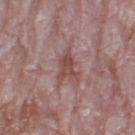No biopsy was performed on this lesion — it was imaged during a full skin examination and was not determined to be concerning. Automated tile analysis of the lesion measured a lesion area of about 5 mm², an eccentricity of roughly 0.85, and a symmetry-axis asymmetry near 0.45. It also reported an average lesion color of about L≈47 a*≈22 b*≈21 (CIELAB) and roughly 9 lightness units darker than nearby skin. The analysis additionally found a border-irregularity index near 5/10, a within-lesion color-variation index near 3/10, and radial color variation of about 1. It also reported a nevus-likeness score of about 0/100 and a lesion-detection confidence of about 70/100. Measured at roughly 3.5 mm in maximum diameter. A female subject aged around 65. A close-up tile cropped from a whole-body skin photograph, about 15 mm across. The lesion is on the leg.